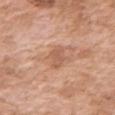No biopsy was performed on this lesion — it was imaged during a full skin examination and was not determined to be concerning. A 15 mm close-up extracted from a 3D total-body photography capture. The patient is a female in their mid- to late 70s. The lesion is located on the right upper arm.